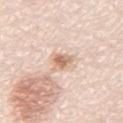Notes:
* notes — total-body-photography surveillance lesion; no biopsy
* patient — male, aged around 80
* size — about 3.5 mm
* lighting — white-light
* acquisition — ~15 mm tile from a whole-body skin photo
* site — the abdomen